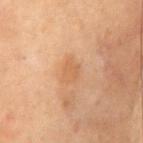| field | value |
|---|---|
| biopsy status | no biopsy performed (imaged during a skin exam) |
| image | total-body-photography crop, ~15 mm field of view |
| illumination | cross-polarized |
| size | ≈3 mm |
| anatomic site | the chest |
| patient | male, aged 68–72 |